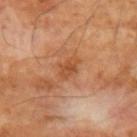{
  "biopsy_status": "not biopsied; imaged during a skin examination",
  "automated_metrics": {
    "area_mm2_approx": 4.5,
    "eccentricity": 0.75,
    "shape_asymmetry": 0.25,
    "cielab_L": 49,
    "cielab_a": 26,
    "cielab_b": 37,
    "vs_skin_darker_L": 8.0,
    "color_variation_0_10": 2.5,
    "peripheral_color_asymmetry": 0.5,
    "nevus_likeness_0_100": 0,
    "lesion_detection_confidence_0_100": 100
  },
  "lighting": "cross-polarized",
  "image": {
    "source": "total-body photography crop",
    "field_of_view_mm": 15
  },
  "lesion_size": {
    "long_diameter_mm_approx": 3.0
  },
  "site": "left upper arm",
  "patient": {
    "sex": "male",
    "age_approx": 70
  }
}